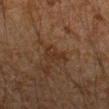Impression: Recorded during total-body skin imaging; not selected for excision or biopsy. Context: A 15 mm crop from a total-body photograph taken for skin-cancer surveillance. Located on the right forearm. A male subject aged around 45. Captured under cross-polarized illumination. The lesion-visualizer software estimated a normalized border contrast of about 6.5.